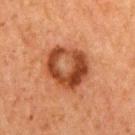{"biopsy_status": "not biopsied; imaged during a skin examination", "image": {"source": "total-body photography crop", "field_of_view_mm": 15}, "patient": {"sex": "female", "age_approx": 60}, "site": "right upper arm"}